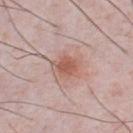notes=catalogued during a skin exam; not biopsied | image=15 mm crop, total-body photography | site=the chest | patient=male, aged approximately 35 | lesion diameter=≈3.5 mm | illumination=white-light.